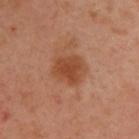  biopsy_status: not biopsied; imaged during a skin examination
  site: upper back
  patient:
    sex: male
    age_approx: 40
  image:
    source: total-body photography crop
    field_of_view_mm: 15